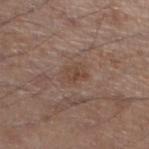notes = imaged on a skin check; not biopsied
lesion size = ~3 mm (longest diameter)
anatomic site = the left lower leg
patient = male, aged 53 to 57
illumination = white-light
imaging modality = 15 mm crop, total-body photography
image-analysis metrics = a lesion area of about 5 mm² and a shape eccentricity near 0.7; a mean CIELAB color near L≈45 a*≈17 b*≈26, roughly 6 lightness units darker than nearby skin, and a normalized border contrast of about 5.5; border irregularity of about 3 on a 0–10 scale and a color-variation rating of about 3/10; a nevus-likeness score of about 0/100 and a detector confidence of about 100 out of 100 that the crop contains a lesion